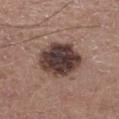Context: Located on the left thigh. A male patient, in their mid-50s. Imaged with white-light lighting. Measured at roughly 5.5 mm in maximum diameter. A 15 mm close-up extracted from a 3D total-body photography capture. The total-body-photography lesion software estimated a lesion area of about 20 mm², an eccentricity of roughly 0.5, and a shape-asymmetry score of about 0.15 (0 = symmetric). The analysis additionally found internal color variation of about 6 on a 0–10 scale and a peripheral color-asymmetry measure near 1.5. It also reported a nevus-likeness score of about 5/100 and a detector confidence of about 100 out of 100 that the crop contains a lesion.